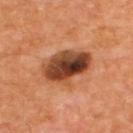This lesion was catalogued during total-body skin photography and was not selected for biopsy. About 5.5 mm across. The tile uses cross-polarized illumination. A lesion tile, about 15 mm wide, cut from a 3D total-body photograph. The patient is a male aged 53–57. Located on the upper back. The lesion-visualizer software estimated a classifier nevus-likeness of about 10/100 and a detector confidence of about 100 out of 100 that the crop contains a lesion.